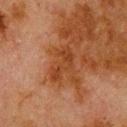Part of a total-body skin-imaging series; this lesion was reviewed on a skin check and was not flagged for biopsy.
Measured at roughly 4.5 mm in maximum diameter.
The lesion-visualizer software estimated roughly 7 lightness units darker than nearby skin and a normalized border contrast of about 7. The software also gave a within-lesion color-variation index near 2/10 and radial color variation of about 0.5. It also reported an automated nevus-likeness rating near 0 out of 100 and a lesion-detection confidence of about 100/100.
A 15 mm close-up extracted from a 3D total-body photography capture.
From the upper back.
A male patient, roughly 80 years of age.
Captured under cross-polarized illumination.